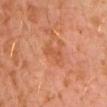The lesion was tiled from a total-body skin photograph and was not biopsied. A male subject approximately 30 years of age. Located on the left upper arm. A 15 mm close-up extracted from a 3D total-body photography capture. Approximately 2.5 mm at its widest.